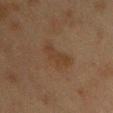Assessment:
Imaged during a routine full-body skin examination; the lesion was not biopsied and no histopathology is available.